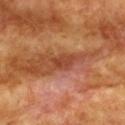Findings:
- notes — total-body-photography surveillance lesion; no biopsy
- tile lighting — cross-polarized illumination
- size — ~2.5 mm (longest diameter)
- patient — female, roughly 75 years of age
- image — ~15 mm tile from a whole-body skin photo
- location — the front of the torso